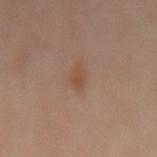Q: Was a biopsy performed?
A: catalogued during a skin exam; not biopsied
Q: How was this image acquired?
A: ~15 mm tile from a whole-body skin photo
Q: Who is the patient?
A: female, aged around 50
Q: Illumination type?
A: cross-polarized
Q: Lesion location?
A: the mid back